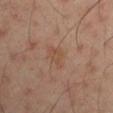{"biopsy_status": "not biopsied; imaged during a skin examination", "image": {"source": "total-body photography crop", "field_of_view_mm": 15}, "site": "left arm", "automated_metrics": {"area_mm2_approx": 4.5, "eccentricity": 0.75, "shape_asymmetry": 0.4, "cielab_L": 48, "cielab_a": 19, "cielab_b": 28, "vs_skin_contrast_norm": 5.0}, "lighting": "cross-polarized", "lesion_size": {"long_diameter_mm_approx": 3.0}, "patient": {"sex": "male", "age_approx": 50}}